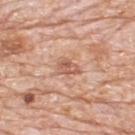Q: What is the anatomic site?
A: the back
Q: Who is the patient?
A: male, approximately 80 years of age
Q: What did automated image analysis measure?
A: a nevus-likeness score of about 0/100
Q: What lighting was used for the tile?
A: white-light
Q: What kind of image is this?
A: total-body-photography crop, ~15 mm field of view